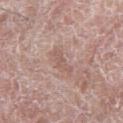Impression:
No biopsy was performed on this lesion — it was imaged during a full skin examination and was not determined to be concerning.
Image and clinical context:
A region of skin cropped from a whole-body photographic capture, roughly 15 mm wide. This is a white-light tile. From the right thigh. The subject is a male aged around 80.